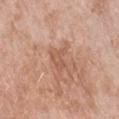Background: The tile uses white-light illumination. A roughly 15 mm field-of-view crop from a total-body skin photograph. On the left forearm. A female subject aged 68 to 72. Longest diameter approximately 3 mm.